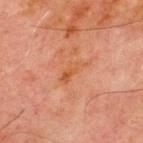No biopsy was performed on this lesion — it was imaged during a full skin examination and was not determined to be concerning. Cropped from a total-body skin-imaging series; the visible field is about 15 mm. Located on the chest. The patient is a male aged around 70. Imaged with cross-polarized lighting. Measured at roughly 3.5 mm in maximum diameter.